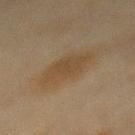Q: Was this lesion biopsied?
A: total-body-photography surveillance lesion; no biopsy